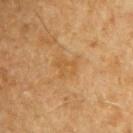<lesion>
  <biopsy_status>not biopsied; imaged during a skin examination</biopsy_status>
  <lighting>cross-polarized</lighting>
  <patient>
    <sex>male</sex>
    <age_approx>60</age_approx>
  </patient>
  <site>chest</site>
  <image>
    <source>total-body photography crop</source>
    <field_of_view_mm>15</field_of_view_mm>
  </image>
  <automated_metrics>
    <border_irregularity_0_10>3.5</border_irregularity_0_10>
    <color_variation_0_10>2.0</color_variation_0_10>
  </automated_metrics>
  <lesion_size>
    <long_diameter_mm_approx>3.0</long_diameter_mm_approx>
  </lesion_size>
</lesion>